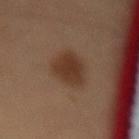Imaged with cross-polarized lighting.
A male subject about 60 years old.
A lesion tile, about 15 mm wide, cut from a 3D total-body photograph.
On the front of the torso.
About 4.5 mm across.